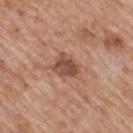{
  "biopsy_status": "not biopsied; imaged during a skin examination",
  "site": "mid back",
  "lighting": "white-light",
  "lesion_size": {
    "long_diameter_mm_approx": 3.0
  },
  "patient": {
    "sex": "male",
    "age_approx": 60
  },
  "image": {
    "source": "total-body photography crop",
    "field_of_view_mm": 15
  }
}